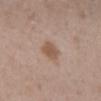Clinical summary:
A 15 mm close-up extracted from a 3D total-body photography capture. From the mid back. Imaged with white-light lighting. The lesion-visualizer software estimated internal color variation of about 2.5 on a 0–10 scale and a peripheral color-asymmetry measure near 1. The software also gave a detector confidence of about 100 out of 100 that the crop contains a lesion. A female subject about 30 years old. Measured at roughly 2.5 mm in maximum diameter.